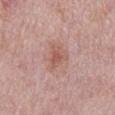Notes:
- notes · catalogued during a skin exam; not biopsied
- image · 15 mm crop, total-body photography
- body site · the mid back
- subject · male, approximately 75 years of age